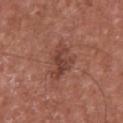Recorded during total-body skin imaging; not selected for excision or biopsy.
A male subject, in their mid- to late 60s.
Approximately 5 mm at its widest.
The lesion is located on the chest.
The tile uses white-light illumination.
Cropped from a total-body skin-imaging series; the visible field is about 15 mm.